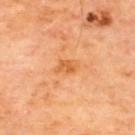{"biopsy_status": "not biopsied; imaged during a skin examination"}